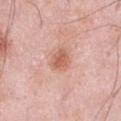<tbp_lesion>
  <biopsy_status>not biopsied; imaged during a skin examination</biopsy_status>
  <patient>
    <sex>male</sex>
    <age_approx>55</age_approx>
  </patient>
  <lighting>white-light</lighting>
  <image>
    <source>total-body photography crop</source>
    <field_of_view_mm>15</field_of_view_mm>
  </image>
  <site>front of the torso</site>
  <lesion_size>
    <long_diameter_mm_approx>3.0</long_diameter_mm_approx>
  </lesion_size>
</tbp_lesion>